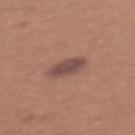follow-up: no biopsy performed (imaged during a skin exam)
acquisition: total-body-photography crop, ~15 mm field of view
anatomic site: the chest
tile lighting: white-light illumination
automated metrics: a lesion area of about 6.5 mm², an outline eccentricity of about 0.8 (0 = round, 1 = elongated), and a symmetry-axis asymmetry near 0.15; a lesion color around L≈47 a*≈19 b*≈22 in CIELAB, roughly 10 lightness units darker than nearby skin, and a normalized lesion–skin contrast near 9; a border-irregularity rating of about 2/10 and internal color variation of about 2 on a 0–10 scale; an automated nevus-likeness rating near 70 out of 100 and a detector confidence of about 100 out of 100 that the crop contains a lesion
subject: female, aged 33–37
size: about 3.5 mm